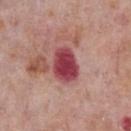<case>
<biopsy_status>not biopsied; imaged during a skin examination</biopsy_status>
<lesion_size>
  <long_diameter_mm_approx>4.5</long_diameter_mm_approx>
</lesion_size>
<patient>
  <sex>male</sex>
  <age_approx>75</age_approx>
</patient>
<image>
  <source>total-body photography crop</source>
  <field_of_view_mm>15</field_of_view_mm>
</image>
<site>chest</site>
<lighting>white-light</lighting>
</case>